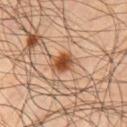The lesion was photographed on a routine skin check and not biopsied; there is no pathology result.
Automated tile analysis of the lesion measured a shape eccentricity near 0.6 and a symmetry-axis asymmetry near 0.2.
The lesion is located on the chest.
Approximately 3 mm at its widest.
A 15 mm close-up extracted from a 3D total-body photography capture.
A male patient about 45 years old.